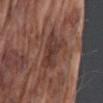The lesion was tiled from a total-body skin photograph and was not biopsied.
Imaged with white-light lighting.
A 15 mm close-up tile from a total-body photography series done for melanoma screening.
A male subject, in their mid-70s.
The lesion is on the left upper arm.